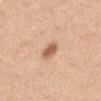No biopsy was performed on this lesion — it was imaged during a full skin examination and was not determined to be concerning.
Cropped from a total-body skin-imaging series; the visible field is about 15 mm.
The subject is a male aged approximately 50.
Captured under white-light illumination.
The lesion's longest dimension is about 2.5 mm.
From the front of the torso.